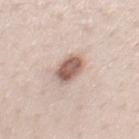No biopsy was performed on this lesion — it was imaged during a full skin examination and was not determined to be concerning. This is a white-light tile. A 15 mm close-up tile from a total-body photography series done for melanoma screening. A male patient aged 38 to 42. The recorded lesion diameter is about 3.5 mm. On the front of the torso.